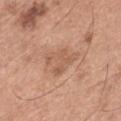workup: catalogued during a skin exam; not biopsied | size: ~4 mm (longest diameter) | image-analysis metrics: a nevus-likeness score of about 0/100 and lesion-presence confidence of about 100/100 | site: the left thigh | imaging modality: 15 mm crop, total-body photography | patient: male, about 65 years old | illumination: white-light.